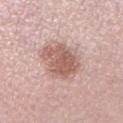Part of a total-body skin-imaging series; this lesion was reviewed on a skin check and was not flagged for biopsy. This is a white-light tile. A lesion tile, about 15 mm wide, cut from a 3D total-body photograph. A male subject, aged 28–32. Measured at roughly 5.5 mm in maximum diameter.